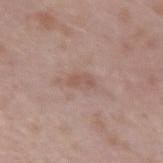• biopsy status — imaged on a skin check; not biopsied
• imaging modality — total-body-photography crop, ~15 mm field of view
• automated metrics — a classifier nevus-likeness of about 0/100 and a detector confidence of about 100 out of 100 that the crop contains a lesion
• illumination — white-light illumination
• location — the left forearm
• patient — male, in their 40s